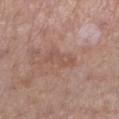workup: catalogued during a skin exam; not biopsied | tile lighting: white-light illumination | patient: female, aged 48 to 52 | lesion diameter: ~4 mm (longest diameter) | imaging modality: ~15 mm tile from a whole-body skin photo | TBP lesion metrics: internal color variation of about 1.5 on a 0–10 scale and peripheral color asymmetry of about 0.5 | site: the leg.